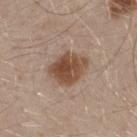Captured during whole-body skin photography for melanoma surveillance; the lesion was not biopsied. The lesion's longest dimension is about 4 mm. The lesion-visualizer software estimated internal color variation of about 5 on a 0–10 scale and a peripheral color-asymmetry measure near 2. The software also gave an automated nevus-likeness rating near 100 out of 100 and lesion-presence confidence of about 100/100. The tile uses white-light illumination. A roughly 15 mm field-of-view crop from a total-body skin photograph. The lesion is on the mid back. A male patient, aged 28 to 32.